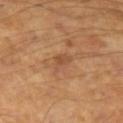{"patient": {"sex": "male", "age_approx": 45}, "lesion_size": {"long_diameter_mm_approx": 3.0}, "lighting": "cross-polarized", "site": "left forearm", "automated_metrics": {"vs_skin_darker_L": 8.0, "vs_skin_contrast_norm": 5.5, "color_variation_0_10": 1.5, "peripheral_color_asymmetry": 0.5}, "image": {"source": "total-body photography crop", "field_of_view_mm": 15}}